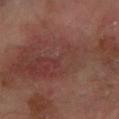* notes — total-body-photography surveillance lesion; no biopsy
* subject — female, roughly 55 years of age
* lighting — cross-polarized
* body site — the arm
* imaging modality — ~15 mm tile from a whole-body skin photo